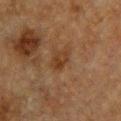workup=no biopsy performed (imaged during a skin exam) | subject=female, in their mid- to late 50s | image source=total-body-photography crop, ~15 mm field of view | automated metrics=an area of roughly 3.5 mm² and a shape eccentricity near 0.75 | lesion size=about 2.5 mm | body site=the chest | tile lighting=cross-polarized.